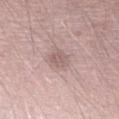biopsy_status: not biopsied; imaged during a skin examination
image:
  source: total-body photography crop
  field_of_view_mm: 15
automated_metrics:
  area_mm2_approx: 4.5
  eccentricity: 0.45
  cielab_L: 59
  cielab_a: 18
  cielab_b: 19
  vs_skin_contrast_norm: 5.5
  border_irregularity_0_10: 1.5
  color_variation_0_10: 2.0
  peripheral_color_asymmetry: 1.0
lighting: white-light
patient:
  sex: male
  age_approx: 30
lesion_size:
  long_diameter_mm_approx: 2.5